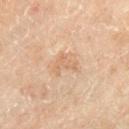No biopsy was performed on this lesion — it was imaged during a full skin examination and was not determined to be concerning.
This image is a 15 mm lesion crop taken from a total-body photograph.
The recorded lesion diameter is about 3 mm.
A male patient roughly 70 years of age.
On the right lower leg.